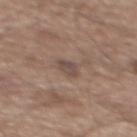image = 15 mm crop, total-body photography | diameter = ≈2.5 mm | patient = male, aged approximately 65 | site = the mid back | illumination = white-light | automated metrics = a footprint of about 3.5 mm², a shape eccentricity near 0.75, and two-axis asymmetry of about 0.3; a border-irregularity rating of about 2.5/10, internal color variation of about 2 on a 0–10 scale, and a peripheral color-asymmetry measure near 1; a classifier nevus-likeness of about 0/100 and a detector confidence of about 100 out of 100 that the crop contains a lesion.